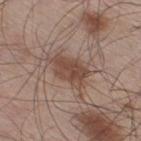Captured during whole-body skin photography for melanoma surveillance; the lesion was not biopsied.
Located on the right thigh.
A close-up tile cropped from a whole-body skin photograph, about 15 mm across.
A male patient in their 60s.
The lesion-visualizer software estimated an area of roughly 10 mm².
The tile uses white-light illumination.